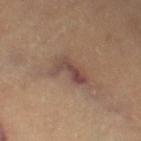A patient in their 60s. A 15 mm close-up extracted from a 3D total-body photography capture. Longest diameter approximately 4.5 mm. The lesion is on the left thigh.